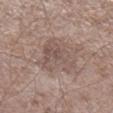A male patient approximately 65 years of age.
Cropped from a total-body skin-imaging series; the visible field is about 15 mm.
An algorithmic analysis of the crop reported a lesion area of about 13 mm², an eccentricity of roughly 0.7, and a symmetry-axis asymmetry near 0.45. The analysis additionally found a mean CIELAB color near L≈52 a*≈15 b*≈22, roughly 7 lightness units darker than nearby skin, and a normalized lesion–skin contrast near 5.5. The analysis additionally found an automated nevus-likeness rating near 10 out of 100 and lesion-presence confidence of about 90/100.
From the right lower leg.
The tile uses white-light illumination.